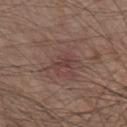tile lighting: white-light illumination | imaging modality: ~15 mm tile from a whole-body skin photo | anatomic site: the chest | subject: male, aged 78 to 82.